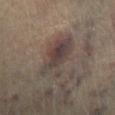Part of a total-body skin-imaging series; this lesion was reviewed on a skin check and was not flagged for biopsy.
The patient is aged approximately 60.
The lesion is on the right lower leg.
A 15 mm crop from a total-body photograph taken for skin-cancer surveillance.
Automated tile analysis of the lesion measured a lesion area of about 15 mm², a shape eccentricity near 0.85, and a symmetry-axis asymmetry near 0.3. The software also gave a nevus-likeness score of about 95/100 and a detector confidence of about 85 out of 100 that the crop contains a lesion.
Imaged with cross-polarized lighting.